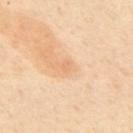Impression:
The lesion was photographed on a routine skin check and not biopsied; there is no pathology result.
Image and clinical context:
Imaged with cross-polarized lighting. A male subject aged 53–57. About 1 mm across. On the mid back. Cropped from a whole-body photographic skin survey; the tile spans about 15 mm.